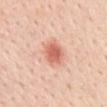Imaged during a routine full-body skin examination; the lesion was not biopsied and no histopathology is available. Imaged with white-light lighting. Cropped from a whole-body photographic skin survey; the tile spans about 15 mm. Located on the mid back. The lesion's longest dimension is about 3.5 mm. A female patient, aged 43 to 47.